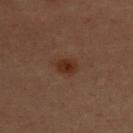  lighting: cross-polarized
  image:
    source: total-body photography crop
    field_of_view_mm: 15
  site: chest
  patient:
    sex: female
    age_approx: 50
  lesion_size:
    long_diameter_mm_approx: 3.0